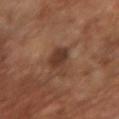Q: Was this lesion biopsied?
A: no biopsy performed (imaged during a skin exam)
Q: Where on the body is the lesion?
A: the right forearm
Q: Lesion size?
A: ≈5 mm
Q: What are the patient's age and sex?
A: female, aged 63 to 67
Q: What is the imaging modality?
A: ~15 mm tile from a whole-body skin photo
Q: Automated lesion metrics?
A: an area of roughly 6.5 mm², a shape eccentricity near 0.9, and a symmetry-axis asymmetry near 0.4
Q: How was the tile lit?
A: cross-polarized illumination